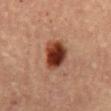diameter: ≈4 mm; image source: 15 mm crop, total-body photography; subject: female, roughly 60 years of age; tile lighting: cross-polarized; site: the abdomen.